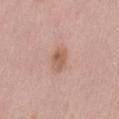The lesion was tiled from a total-body skin photograph and was not biopsied.
A 15 mm crop from a total-body photograph taken for skin-cancer surveillance.
Located on the lower back.
A male subject, about 40 years old.
Approximately 3 mm at its widest.
Captured under white-light illumination.
Automated tile analysis of the lesion measured a mean CIELAB color near L≈58 a*≈21 b*≈29, about 9 CIELAB-L* units darker than the surrounding skin, and a normalized border contrast of about 7. It also reported a border-irregularity index near 1.5/10 and peripheral color asymmetry of about 1. And it measured a detector confidence of about 100 out of 100 that the crop contains a lesion.